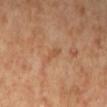Part of a total-body skin-imaging series; this lesion was reviewed on a skin check and was not flagged for biopsy. This is a cross-polarized tile. A roughly 15 mm field-of-view crop from a total-body skin photograph. The lesion's longest dimension is about 2.5 mm. On the left lower leg. A female patient aged 68 to 72. An algorithmic analysis of the crop reported an area of roughly 2.5 mm² and two-axis asymmetry of about 0.4. And it measured a mean CIELAB color near L≈54 a*≈23 b*≈36 and a normalized lesion–skin contrast near 5. And it measured a nevus-likeness score of about 0/100 and a detector confidence of about 100 out of 100 that the crop contains a lesion.